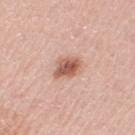biopsy status — catalogued during a skin exam; not biopsied
imaging modality — ~15 mm crop, total-body skin-cancer survey
subject — male, roughly 70 years of age
site — the right thigh